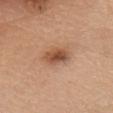workup: catalogued during a skin exam; not biopsied | TBP lesion metrics: a lesion area of about 7 mm² and a shape eccentricity near 0.75; a lesion–skin lightness drop of about 12 and a lesion-to-skin contrast of about 8.5 (normalized; higher = more distinct); a classifier nevus-likeness of about 90/100 and lesion-presence confidence of about 100/100 | tile lighting: white-light illumination | size: about 3.5 mm | image source: 15 mm crop, total-body photography | patient: female, aged 58 to 62 | site: the head or neck.